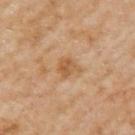Part of a total-body skin-imaging series; this lesion was reviewed on a skin check and was not flagged for biopsy. The lesion-visualizer software estimated an area of roughly 4.5 mm², a shape eccentricity near 0.3, and two-axis asymmetry of about 0.3. And it measured border irregularity of about 3 on a 0–10 scale, a color-variation rating of about 3/10, and peripheral color asymmetry of about 1. Imaged with cross-polarized lighting. The lesion is on the left upper arm. A region of skin cropped from a whole-body photographic capture, roughly 15 mm wide. A female subject, approximately 60 years of age.